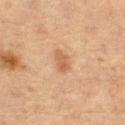Recorded during total-body skin imaging; not selected for excision or biopsy.
A 15 mm close-up extracted from a 3D total-body photography capture.
A female patient, aged 53–57.
The recorded lesion diameter is about 3 mm.
Located on the right thigh.
Captured under cross-polarized illumination.